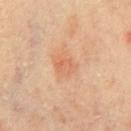Assessment:
This lesion was catalogued during total-body skin photography and was not selected for biopsy.
Clinical summary:
A 15 mm close-up extracted from a 3D total-body photography capture. A male subject in their mid- to late 60s. The lesion is located on the chest.